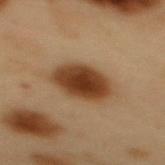workup = no biopsy performed (imaged during a skin exam) | diameter = about 5 mm | image source = ~15 mm tile from a whole-body skin photo | patient = male, aged 53–57 | body site = the mid back.